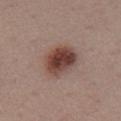Recorded during total-body skin imaging; not selected for excision or biopsy.